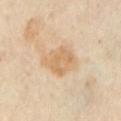Impression:
Imaged during a routine full-body skin examination; the lesion was not biopsied and no histopathology is available.
Context:
This is a cross-polarized tile. A patient aged 53–57. Approximately 4 mm at its widest. Automated image analysis of the tile measured an average lesion color of about L≈66 a*≈16 b*≈36 (CIELAB), roughly 8 lightness units darker than nearby skin, and a normalized lesion–skin contrast near 6. The analysis additionally found a border-irregularity index near 3/10 and peripheral color asymmetry of about 1. The software also gave an automated nevus-likeness rating near 0 out of 100 and a lesion-detection confidence of about 100/100. The lesion is located on the left upper arm. Cropped from a total-body skin-imaging series; the visible field is about 15 mm.